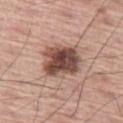| field | value |
|---|---|
| notes | no biopsy performed (imaged during a skin exam) |